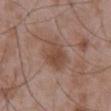* follow-up · total-body-photography surveillance lesion; no biopsy
* tile lighting · white-light illumination
* body site · the mid back
* image · total-body-photography crop, ~15 mm field of view
* size · ~5 mm (longest diameter)
* patient · male, approximately 50 years of age
* TBP lesion metrics · a mean CIELAB color near L≈47 a*≈18 b*≈27, roughly 8 lightness units darker than nearby skin, and a normalized border contrast of about 7; border irregularity of about 3 on a 0–10 scale, a color-variation rating of about 3/10, and a peripheral color-asymmetry measure near 1; a classifier nevus-likeness of about 0/100 and lesion-presence confidence of about 100/100